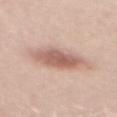workup: no biopsy performed (imaged during a skin exam)
diameter: ~6 mm (longest diameter)
imaging modality: total-body-photography crop, ~15 mm field of view
location: the mid back
patient: female, aged around 40
tile lighting: white-light
automated metrics: a shape eccentricity near 0.8; a lesion color around L≈61 a*≈20 b*≈26 in CIELAB, a lesion–skin lightness drop of about 12, and a normalized border contrast of about 7.5; border irregularity of about 2.5 on a 0–10 scale, a color-variation rating of about 4.5/10, and a peripheral color-asymmetry measure near 1.5